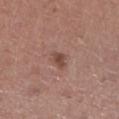workup: no biopsy performed (imaged during a skin exam)
automated lesion analysis: an area of roughly 3 mm² and two-axis asymmetry of about 0.35; a lesion color around L≈46 a*≈21 b*≈25 in CIELAB, a lesion–skin lightness drop of about 10, and a lesion-to-skin contrast of about 8 (normalized; higher = more distinct)
image source: total-body-photography crop, ~15 mm field of view
patient: female, about 65 years old
body site: the right lower leg
size: ≈2.5 mm